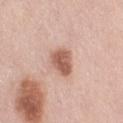illumination: white-light illumination
patient: female, aged approximately 65
size: ≈3 mm
automated lesion analysis: a mean CIELAB color near L≈58 a*≈22 b*≈28 and a normalized border contrast of about 9
image: 15 mm crop, total-body photography
body site: the right thigh